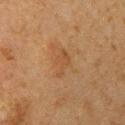Assessment: The lesion was photographed on a routine skin check and not biopsied; there is no pathology result. Background: Automated image analysis of the tile measured about 5 CIELAB-L* units darker than the surrounding skin and a normalized border contrast of about 5. The software also gave a border-irregularity index near 5.5/10, internal color variation of about 1.5 on a 0–10 scale, and peripheral color asymmetry of about 0.5. From the right upper arm. The patient is a female aged around 60. A 15 mm crop from a total-body photograph taken for skin-cancer surveillance. This is a cross-polarized tile.